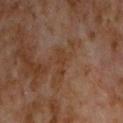- notes · no biopsy performed (imaged during a skin exam)
- location · the chest
- lesion size · ~3.5 mm (longest diameter)
- image · ~15 mm crop, total-body skin-cancer survey
- tile lighting · cross-polarized illumination
- patient · male, aged 58–62